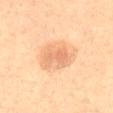notes = catalogued during a skin exam; not biopsied.